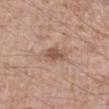Case summary:
– TBP lesion metrics: a footprint of about 4 mm² and a shape eccentricity near 0.5; an average lesion color of about L≈53 a*≈19 b*≈27 (CIELAB), about 10 CIELAB-L* units darker than the surrounding skin, and a normalized lesion–skin contrast near 7; a nevus-likeness score of about 5/100 and a detector confidence of about 100 out of 100 that the crop contains a lesion
– image source: ~15 mm tile from a whole-body skin photo
– body site: the left forearm
– tile lighting: white-light
– subject: male, in their 40s